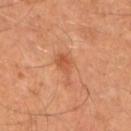Q: Is there a histopathology result?
A: total-body-photography surveillance lesion; no biopsy
Q: Illumination type?
A: cross-polarized illumination
Q: What kind of image is this?
A: total-body-photography crop, ~15 mm field of view
Q: How large is the lesion?
A: about 4 mm
Q: Patient demographics?
A: male, aged 38 to 42
Q: Where on the body is the lesion?
A: the leg